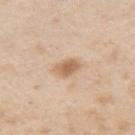Imaged during a routine full-body skin examination; the lesion was not biopsied and no histopathology is available. A 15 mm close-up extracted from a 3D total-body photography capture. A male patient, aged around 45. The lesion's longest dimension is about 2.5 mm. From the left upper arm. Captured under white-light illumination.